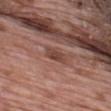The lesion was photographed on a routine skin check and not biopsied; there is no pathology result.
Located on the back.
A male patient, approximately 70 years of age.
A 15 mm close-up extracted from a 3D total-body photography capture.